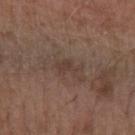Q: How was the tile lit?
A: white-light illumination
Q: How was this image acquired?
A: total-body-photography crop, ~15 mm field of view
Q: What are the patient's age and sex?
A: male, aged approximately 55
Q: What did automated image analysis measure?
A: a footprint of about 3.5 mm², a shape eccentricity near 0.8, and two-axis asymmetry of about 0.45; an automated nevus-likeness rating near 0 out of 100 and a lesion-detection confidence of about 100/100
Q: What is the anatomic site?
A: the left forearm
Q: Lesion size?
A: ~3 mm (longest diameter)